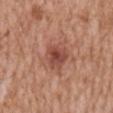A region of skin cropped from a whole-body photographic capture, roughly 15 mm wide.
Automated tile analysis of the lesion measured a lesion area of about 8 mm² and an outline eccentricity of about 0.4 (0 = round, 1 = elongated). The software also gave an average lesion color of about L≈48 a*≈26 b*≈28 (CIELAB) and roughly 10 lightness units darker than nearby skin. It also reported a border-irregularity index near 2.5/10 and a peripheral color-asymmetry measure near 1.5.
A male subject in their 60s.
From the abdomen.
Approximately 3.5 mm at its widest.
The tile uses white-light illumination.